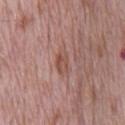Impression: Imaged during a routine full-body skin examination; the lesion was not biopsied and no histopathology is available. Background: Measured at roughly 3 mm in maximum diameter. The lesion is on the chest. A 15 mm close-up tile from a total-body photography series done for melanoma screening. The patient is a male aged around 70. Captured under white-light illumination.